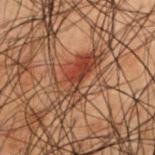Clinical impression:
No biopsy was performed on this lesion — it was imaged during a full skin examination and was not determined to be concerning.
Clinical summary:
Cropped from a whole-body photographic skin survey; the tile spans about 15 mm. From the upper back. The subject is a male aged around 50.